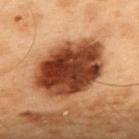<tbp_lesion>
  <biopsy_status>not biopsied; imaged during a skin examination</biopsy_status>
  <lighting>cross-polarized</lighting>
  <patient>
    <sex>male</sex>
    <age_approx>55</age_approx>
  </patient>
  <site>upper back</site>
  <image>
    <source>total-body photography crop</source>
    <field_of_view_mm>15</field_of_view_mm>
  </image>
</tbp_lesion>